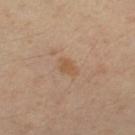Notes:
– biopsy status · total-body-photography surveillance lesion; no biopsy
– body site · the right leg
– image source · 15 mm crop, total-body photography
– subject · female, aged approximately 40